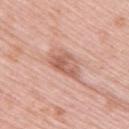This lesion was catalogued during total-body skin photography and was not selected for biopsy. This image is a 15 mm lesion crop taken from a total-body photograph. A female patient, about 65 years old. An algorithmic analysis of the crop reported a border-irregularity index near 3/10, internal color variation of about 5 on a 0–10 scale, and peripheral color asymmetry of about 1.5. The analysis additionally found an automated nevus-likeness rating near 45 out of 100. The lesion is on the upper back. About 4 mm across.